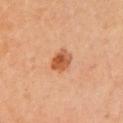Q: Was a biopsy performed?
A: total-body-photography surveillance lesion; no biopsy
Q: Automated lesion metrics?
A: border irregularity of about 1.5 on a 0–10 scale, a within-lesion color-variation index near 5.5/10, and a peripheral color-asymmetry measure near 1.5
Q: How was this image acquired?
A: ~15 mm crop, total-body skin-cancer survey
Q: What is the anatomic site?
A: the left upper arm
Q: Who is the patient?
A: female, aged approximately 35
Q: How was the tile lit?
A: cross-polarized